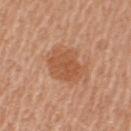Assessment:
Part of a total-body skin-imaging series; this lesion was reviewed on a skin check and was not flagged for biopsy.
Image and clinical context:
Captured under white-light illumination. A roughly 15 mm field-of-view crop from a total-body skin photograph. The lesion is on the left upper arm. The lesion-visualizer software estimated an average lesion color of about L≈54 a*≈24 b*≈36 (CIELAB), about 9 CIELAB-L* units darker than the surrounding skin, and a lesion-to-skin contrast of about 6.5 (normalized; higher = more distinct). The software also gave a border-irregularity rating of about 2.5/10 and a color-variation rating of about 2.5/10. The analysis additionally found a classifier nevus-likeness of about 65/100 and a lesion-detection confidence of about 100/100. A female patient, aged around 55.